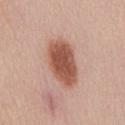Recorded during total-body skin imaging; not selected for excision or biopsy.
The lesion is located on the front of the torso.
The tile uses white-light illumination.
A male subject, in their mid- to late 50s.
A 15 mm crop from a total-body photograph taken for skin-cancer surveillance.
Measured at roughly 6 mm in maximum diameter.